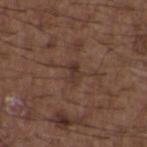Captured during whole-body skin photography for melanoma surveillance; the lesion was not biopsied.
A male subject, about 50 years old.
This image is a 15 mm lesion crop taken from a total-body photograph.
The tile uses white-light illumination.
The lesion is on the upper back.
About 2.5 mm across.